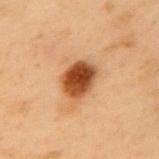Image and clinical context:
Located on the back. The lesion-visualizer software estimated an area of roughly 11 mm², an outline eccentricity of about 0.65 (0 = round, 1 = elongated), and a symmetry-axis asymmetry near 0.15. A roughly 15 mm field-of-view crop from a total-body skin photograph. A male subject aged 53–57. This is a cross-polarized tile. The recorded lesion diameter is about 4 mm.